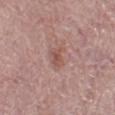| field | value |
|---|---|
| biopsy status | total-body-photography surveillance lesion; no biopsy |
| patient | female, about 65 years old |
| location | the leg |
| lesion size | about 3 mm |
| image | total-body-photography crop, ~15 mm field of view |